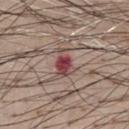Impression:
The lesion was tiled from a total-body skin photograph and was not biopsied.
Context:
About 3.5 mm across. Located on the chest. This is a white-light tile. The total-body-photography lesion software estimated a lesion area of about 5.5 mm², an eccentricity of roughly 0.75, and two-axis asymmetry of about 0.4. The analysis additionally found a mean CIELAB color near L≈44 a*≈24 b*≈20, roughly 13 lightness units darker than nearby skin, and a normalized border contrast of about 10. The software also gave a nevus-likeness score of about 0/100 and a lesion-detection confidence of about 100/100. The subject is a male in their mid- to late 50s. This image is a 15 mm lesion crop taken from a total-body photograph.